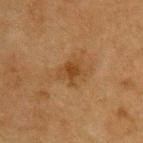Notes:
• biopsy status — imaged on a skin check; not biopsied
• lighting — cross-polarized illumination
• body site — the upper back
• diameter — about 3 mm
• subject — male, aged around 75
• image source — ~15 mm tile from a whole-body skin photo